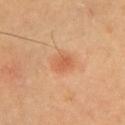biopsy_status: not biopsied; imaged during a skin examination
lighting: cross-polarized
lesion_size:
  long_diameter_mm_approx: 3.0
site: chest
image:
  source: total-body photography crop
  field_of_view_mm: 15